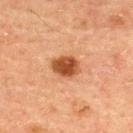Image and clinical context:
The lesion's longest dimension is about 3.5 mm. The subject is a male aged approximately 60. Cropped from a total-body skin-imaging series; the visible field is about 15 mm. Automated tile analysis of the lesion measured border irregularity of about 1.5 on a 0–10 scale and radial color variation of about 1. The analysis additionally found an automated nevus-likeness rating near 100 out of 100 and a detector confidence of about 100 out of 100 that the crop contains a lesion. The tile uses cross-polarized illumination.